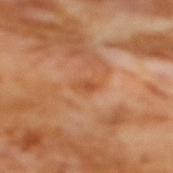follow-up = imaged on a skin check; not biopsied
acquisition = ~15 mm tile from a whole-body skin photo
site = the back
patient = female, aged 53 to 57
TBP lesion metrics = a shape eccentricity near 0.85; a lesion color around L≈53 a*≈27 b*≈40 in CIELAB and about 7 CIELAB-L* units darker than the surrounding skin; a border-irregularity index near 2/10, a color-variation rating of about 0.5/10, and radial color variation of about 0; a classifier nevus-likeness of about 0/100 and a detector confidence of about 100 out of 100 that the crop contains a lesion
tile lighting = cross-polarized illumination